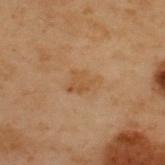Impression: The lesion was photographed on a routine skin check and not biopsied; there is no pathology result. Background: The lesion is located on the upper back. A roughly 15 mm field-of-view crop from a total-body skin photograph. The patient is a male aged 53–57.